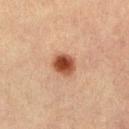Impression:
This lesion was catalogued during total-body skin photography and was not selected for biopsy.
Image and clinical context:
A 15 mm close-up extracted from a 3D total-body photography capture. The tile uses cross-polarized illumination. The lesion is on the left thigh. A female subject, approximately 65 years of age. An algorithmic analysis of the crop reported a lesion area of about 6.5 mm², an outline eccentricity of about 0.55 (0 = round, 1 = elongated), and a symmetry-axis asymmetry near 0.15.